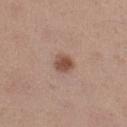notes: imaged on a skin check; not biopsied
size: ≈2.5 mm
image: total-body-photography crop, ~15 mm field of view
illumination: white-light illumination
body site: the leg
subject: female, in their mid-40s